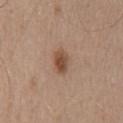<lesion>
<biopsy_status>not biopsied; imaged during a skin examination</biopsy_status>
<lesion_size>
  <long_diameter_mm_approx>3.0</long_diameter_mm_approx>
</lesion_size>
<patient>
  <sex>male</sex>
  <age_approx>50</age_approx>
</patient>
<site>mid back</site>
<image>
  <source>total-body photography crop</source>
  <field_of_view_mm>15</field_of_view_mm>
</image>
</lesion>